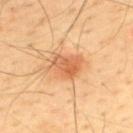lighting: cross-polarized illumination | image source: total-body-photography crop, ~15 mm field of view | subject: male, aged approximately 45 | site: the mid back.